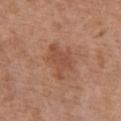Clinical impression: Recorded during total-body skin imaging; not selected for excision or biopsy. Acquisition and patient details: This image is a 15 mm lesion crop taken from a total-body photograph. A female patient, aged approximately 75. The tile uses white-light illumination. The total-body-photography lesion software estimated an automated nevus-likeness rating near 0 out of 100 and a lesion-detection confidence of about 100/100. Located on the chest.